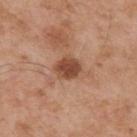{"biopsy_status": "not biopsied; imaged during a skin examination", "lesion_size": {"long_diameter_mm_approx": 3.0}, "patient": {"sex": "male", "age_approx": 55}, "lighting": "white-light", "automated_metrics": {"cielab_L": 47, "cielab_a": 23, "cielab_b": 31, "border_irregularity_0_10": 2.0, "color_variation_0_10": 2.5}, "site": "upper back", "image": {"source": "total-body photography crop", "field_of_view_mm": 15}}